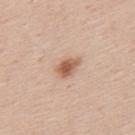The lesion was tiled from a total-body skin photograph and was not biopsied. Measured at roughly 3 mm in maximum diameter. The lesion is on the back. A male subject aged approximately 45. Imaged with white-light lighting. A close-up tile cropped from a whole-body skin photograph, about 15 mm across.